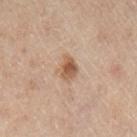follow-up: imaged on a skin check; not biopsied
anatomic site: the leg
imaging modality: ~15 mm tile from a whole-body skin photo
lesion size: ≈2.5 mm
subject: female, aged 53–57